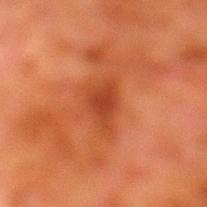follow-up — total-body-photography surveillance lesion; no biopsy | lighting — cross-polarized | acquisition — ~15 mm crop, total-body skin-cancer survey | lesion diameter — about 4 mm | patient — male, roughly 80 years of age | body site — the left lower leg.